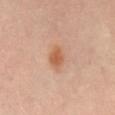Part of a total-body skin-imaging series; this lesion was reviewed on a skin check and was not flagged for biopsy.
On the mid back.
Longest diameter approximately 3.5 mm.
A 15 mm close-up extracted from a 3D total-body photography capture.
The patient is a female roughly 65 years of age.
An algorithmic analysis of the crop reported a shape eccentricity near 0.75 and a shape-asymmetry score of about 0.15 (0 = symmetric). The analysis additionally found a nevus-likeness score of about 90/100.
The tile uses cross-polarized illumination.